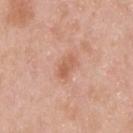The lesion was tiled from a total-body skin photograph and was not biopsied. On the upper back. The subject is a male aged approximately 45. Imaged with white-light lighting. Cropped from a whole-body photographic skin survey; the tile spans about 15 mm.